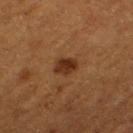{"biopsy_status": "not biopsied; imaged during a skin examination", "site": "left thigh", "lesion_size": {"long_diameter_mm_approx": 2.5}, "patient": {"sex": "female", "age_approx": 50}, "image": {"source": "total-body photography crop", "field_of_view_mm": 15}}